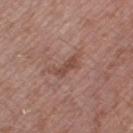Clinical impression: This lesion was catalogued during total-body skin photography and was not selected for biopsy. Background: About 4.5 mm across. The lesion is on the right thigh. The tile uses white-light illumination. A roughly 15 mm field-of-view crop from a total-body skin photograph. Automated image analysis of the tile measured a normalized lesion–skin contrast near 6. It also reported border irregularity of about 6 on a 0–10 scale, a within-lesion color-variation index near 1.5/10, and radial color variation of about 0.5. A male patient, approximately 45 years of age.